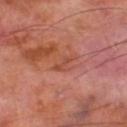Part of a total-body skin-imaging series; this lesion was reviewed on a skin check and was not flagged for biopsy.
The subject is a male in their 70s.
About 2.5 mm across.
The lesion is on the left thigh.
A 15 mm close-up extracted from a 3D total-body photography capture.
The tile uses cross-polarized illumination.